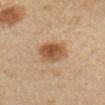{
  "biopsy_status": "not biopsied; imaged during a skin examination",
  "lighting": "cross-polarized",
  "patient": {
    "sex": "female",
    "age_approx": 45
  },
  "image": {
    "source": "total-body photography crop",
    "field_of_view_mm": 15
  },
  "lesion_size": {
    "long_diameter_mm_approx": 4.0
  },
  "site": "left forearm"
}